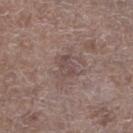workup: catalogued during a skin exam; not biopsied
lesion diameter: ≈2.5 mm
imaging modality: ~15 mm tile from a whole-body skin photo
image-analysis metrics: an average lesion color of about L≈46 a*≈16 b*≈19 (CIELAB), a lesion–skin lightness drop of about 6, and a normalized lesion–skin contrast near 5; internal color variation of about 1 on a 0–10 scale and peripheral color asymmetry of about 0.5; a nevus-likeness score of about 0/100
subject: male, aged 68 to 72
location: the leg
lighting: white-light